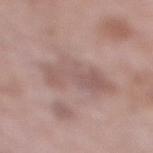{
  "biopsy_status": "not biopsied; imaged during a skin examination",
  "lighting": "white-light",
  "lesion_size": {
    "long_diameter_mm_approx": 8.0
  },
  "site": "right lower leg",
  "patient": {
    "sex": "female",
    "age_approx": 70
  },
  "image": {
    "source": "total-body photography crop",
    "field_of_view_mm": 15
  }
}